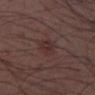Notes:
- workup · no biopsy performed (imaged during a skin exam)
- patient · male, approximately 30 years of age
- site · the front of the torso
- illumination · white-light illumination
- imaging modality · ~15 mm tile from a whole-body skin photo